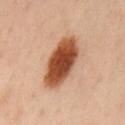{
  "biopsy_status": "not biopsied; imaged during a skin examination",
  "image": {
    "source": "total-body photography crop",
    "field_of_view_mm": 15
  },
  "site": "mid back",
  "automated_metrics": {
    "cielab_L": 51,
    "cielab_a": 26,
    "cielab_b": 35,
    "vs_skin_darker_L": 20.0,
    "vs_skin_contrast_norm": 13.0,
    "nevus_likeness_0_100": 100,
    "lesion_detection_confidence_0_100": 100
  },
  "patient": {
    "sex": "male",
    "age_approx": 55
  }
}